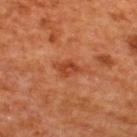Clinical impression:
The lesion was tiled from a total-body skin photograph and was not biopsied.
Background:
Longest diameter approximately 3 mm. The patient is a male about 65 years old. Located on the back. A close-up tile cropped from a whole-body skin photograph, about 15 mm across. This is a cross-polarized tile.